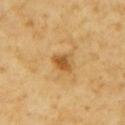workup: imaged on a skin check; not biopsied